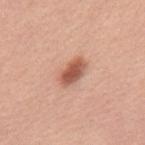Context:
Automated tile analysis of the lesion measured a lesion color around L≈56 a*≈25 b*≈31 in CIELAB and roughly 15 lightness units darker than nearby skin. The analysis additionally found a border-irregularity rating of about 2/10, a within-lesion color-variation index near 3.5/10, and radial color variation of about 1. Captured under white-light illumination. A lesion tile, about 15 mm wide, cut from a 3D total-body photograph. The patient is a female approximately 55 years of age. The lesion is on the back.